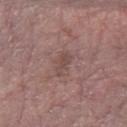Image and clinical context: A roughly 15 mm field-of-view crop from a total-body skin photograph. The total-body-photography lesion software estimated a lesion area of about 4 mm², an eccentricity of roughly 0.85, and a symmetry-axis asymmetry near 0.3. The analysis additionally found an automated nevus-likeness rating near 0 out of 100 and lesion-presence confidence of about 100/100. The lesion is on the left forearm. A female subject about 65 years old. This is a white-light tile.